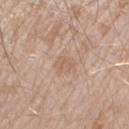biopsy status: catalogued during a skin exam; not biopsied | anatomic site: the arm | patient: male, approximately 60 years of age | tile lighting: white-light illumination | image-analysis metrics: a border-irregularity index near 2.5/10, a within-lesion color-variation index near 2/10, and peripheral color asymmetry of about 0.5 | imaging modality: 15 mm crop, total-body photography | lesion diameter: ≈2.5 mm.